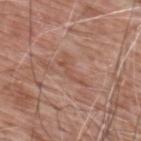Notes:
– notes · total-body-photography surveillance lesion; no biopsy
– site · the upper back
– imaging modality · total-body-photography crop, ~15 mm field of view
– tile lighting · white-light
– size · ~4 mm (longest diameter)
– patient · male, aged approximately 60